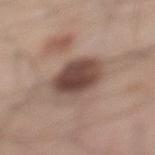The lesion was tiled from a total-body skin photograph and was not biopsied.
The patient is a male approximately 25 years of age.
This is a white-light tile.
On the left lower leg.
A 15 mm close-up tile from a total-body photography series done for melanoma screening.